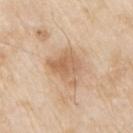Impression:
Imaged during a routine full-body skin examination; the lesion was not biopsied and no histopathology is available.
Image and clinical context:
This image is a 15 mm lesion crop taken from a total-body photograph. A male patient aged 78–82. Imaged with white-light lighting. Located on the left upper arm. The recorded lesion diameter is about 4.5 mm.